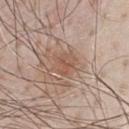<lesion>
  <biopsy_status>not biopsied; imaged during a skin examination</biopsy_status>
  <lighting>white-light</lighting>
  <image>
    <source>total-body photography crop</source>
    <field_of_view_mm>15</field_of_view_mm>
  </image>
  <lesion_size>
    <long_diameter_mm_approx>3.0</long_diameter_mm_approx>
  </lesion_size>
  <patient>
    <sex>male</sex>
    <age_approx>80</age_approx>
  </patient>
  <site>chest</site>
</lesion>